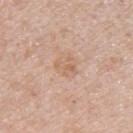Imaged during a routine full-body skin examination; the lesion was not biopsied and no histopathology is available. A male patient, aged 48–52. On the upper back. A close-up tile cropped from a whole-body skin photograph, about 15 mm across.